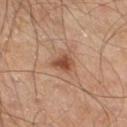| field | value |
|---|---|
| notes | catalogued during a skin exam; not biopsied |
| lighting | cross-polarized illumination |
| automated metrics | a lesion area of about 5 mm² and an eccentricity of roughly 0.5; a within-lesion color-variation index near 3.5/10 and a peripheral color-asymmetry measure near 1 |
| location | the right thigh |
| lesion diameter | ~2.5 mm (longest diameter) |
| image | ~15 mm tile from a whole-body skin photo |
| patient | male, approximately 60 years of age |